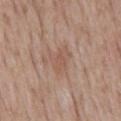Q: What is the imaging modality?
A: total-body-photography crop, ~15 mm field of view
Q: Lesion location?
A: the mid back
Q: How large is the lesion?
A: ~3.5 mm (longest diameter)
Q: How was the tile lit?
A: white-light illumination
Q: Who is the patient?
A: male, in their mid- to late 70s